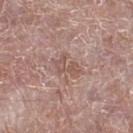| feature | finding |
|---|---|
| biopsy status | no biopsy performed (imaged during a skin exam) |
| patient | male, in their mid- to late 60s |
| image source | ~15 mm crop, total-body skin-cancer survey |
| tile lighting | white-light |
| anatomic site | the left lower leg |